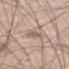Notes:
- subject · male, approximately 45 years of age
- tile lighting · white-light
- anatomic site · the left lower leg
- automated lesion analysis · an average lesion color of about L≈59 a*≈18 b*≈25 (CIELAB), roughly 9 lightness units darker than nearby skin, and a normalized lesion–skin contrast near 6; a classifier nevus-likeness of about 95/100 and a lesion-detection confidence of about 95/100
- lesion diameter · ~3.5 mm (longest diameter)
- acquisition · total-body-photography crop, ~15 mm field of view